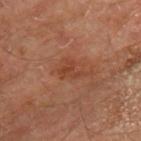Findings:
– workup: imaged on a skin check; not biopsied
– patient: male, aged around 65
– image source: 15 mm crop, total-body photography
– site: the upper back
– TBP lesion metrics: an eccentricity of roughly 0.7 and two-axis asymmetry of about 0.55; an average lesion color of about L≈41 a*≈25 b*≈32 (CIELAB), about 7 CIELAB-L* units darker than the surrounding skin, and a normalized border contrast of about 6; border irregularity of about 6 on a 0–10 scale, a color-variation rating of about 2.5/10, and a peripheral color-asymmetry measure near 1
– lighting: cross-polarized illumination
– size: ~3.5 mm (longest diameter)